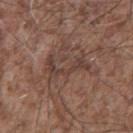Imaged during a routine full-body skin examination; the lesion was not biopsied and no histopathology is available.
Approximately 5 mm at its widest.
A 15 mm crop from a total-body photograph taken for skin-cancer surveillance.
This is a white-light tile.
The lesion is on the mid back.
The patient is a male aged 53 to 57.